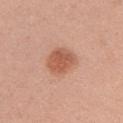Captured during whole-body skin photography for melanoma surveillance; the lesion was not biopsied. Approximately 3.5 mm at its widest. Located on the left upper arm. The patient is a female aged 33–37. A lesion tile, about 15 mm wide, cut from a 3D total-body photograph. The tile uses white-light illumination.